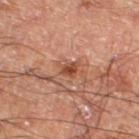<tbp_lesion>
<biopsy_status>not biopsied; imaged during a skin examination</biopsy_status>
<site>right thigh</site>
<lesion_size>
  <long_diameter_mm_approx>2.0</long_diameter_mm_approx>
</lesion_size>
<patient>
  <sex>male</sex>
  <age_approx>60</age_approx>
</patient>
<image>
  <source>total-body photography crop</source>
  <field_of_view_mm>15</field_of_view_mm>
</image>
<automated_metrics>
  <area_mm2_approx>2.5</area_mm2_approx>
  <eccentricity>0.65</eccentricity>
  <shape_asymmetry>0.4</shape_asymmetry>
  <cielab_L>42</cielab_L>
  <cielab_a>25</cielab_a>
  <cielab_b>29</cielab_b>
  <vs_skin_darker_L>11.0</vs_skin_darker_L>
  <vs_skin_contrast_norm>8.5</vs_skin_contrast_norm>
  <nevus_likeness_0_100>45</nevus_likeness_0_100>
  <lesion_detection_confidence_0_100>100</lesion_detection_confidence_0_100>
</automated_metrics>
<lighting>cross-polarized</lighting>
</tbp_lesion>